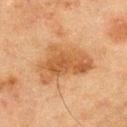Context: A male subject approximately 70 years of age. Cropped from a whole-body photographic skin survey; the tile spans about 15 mm. From the upper back. Automated tile analysis of the lesion measured internal color variation of about 4.5 on a 0–10 scale and a peripheral color-asymmetry measure near 1.5. It also reported a nevus-likeness score of about 55/100.